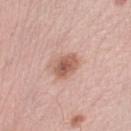The lesion was photographed on a routine skin check and not biopsied; there is no pathology result.
A region of skin cropped from a whole-body photographic capture, roughly 15 mm wide.
About 3.5 mm across.
The tile uses white-light illumination.
From the left lower leg.
A female patient, aged 63 to 67.
Automated image analysis of the tile measured a lesion area of about 7 mm², an outline eccentricity of about 0.65 (0 = round, 1 = elongated), and a shape-asymmetry score of about 0.2 (0 = symmetric).